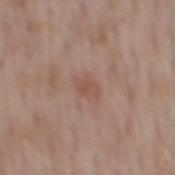{
  "lighting": "white-light",
  "site": "back",
  "patient": {
    "sex": "male",
    "age_approx": 55
  },
  "lesion_size": {
    "long_diameter_mm_approx": 2.5
  },
  "image": {
    "source": "total-body photography crop",
    "field_of_view_mm": 15
  }
}